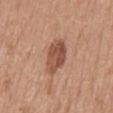Imaged during a routine full-body skin examination; the lesion was not biopsied and no histopathology is available. The patient is a female in their 40s. The tile uses white-light illumination. A 15 mm close-up extracted from a 3D total-body photography capture. The lesion is on the mid back.